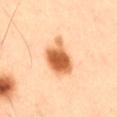notes = total-body-photography surveillance lesion; no biopsy
body site = the right thigh
subject = male, in their 50s
image = 15 mm crop, total-body photography
diameter = about 5 mm